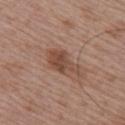biopsy status — imaged on a skin check; not biopsied
size — ~5 mm (longest diameter)
patient — male, in their mid- to late 60s
illumination — white-light
site — the arm
automated lesion analysis — a lesion color around L≈47 a*≈20 b*≈27 in CIELAB, a lesion–skin lightness drop of about 10, and a normalized border contrast of about 7.5; border irregularity of about 4 on a 0–10 scale, a color-variation rating of about 4/10, and radial color variation of about 1
image — ~15 mm crop, total-body skin-cancer survey